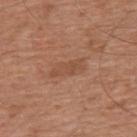<case>
<patient>
  <sex>male</sex>
  <age_approx>65</age_approx>
</patient>
<lighting>white-light</lighting>
<site>upper back</site>
<image>
  <source>total-body photography crop</source>
  <field_of_view_mm>15</field_of_view_mm>
</image>
<lesion_size>
  <long_diameter_mm_approx>4.5</long_diameter_mm_approx>
</lesion_size>
</case>